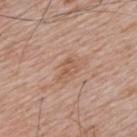Assessment:
Captured during whole-body skin photography for melanoma surveillance; the lesion was not biopsied.